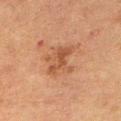follow-up: catalogued during a skin exam; not biopsied
lesion diameter: ≈4.5 mm
tile lighting: cross-polarized
imaging modality: ~15 mm tile from a whole-body skin photo
location: the abdomen
patient: female, about 50 years old
image-analysis metrics: a lesion area of about 11 mm² and an eccentricity of roughly 0.65; a lesion color around L≈44 a*≈20 b*≈30 in CIELAB, about 7 CIELAB-L* units darker than the surrounding skin, and a normalized border contrast of about 6; a border-irregularity index near 4.5/10, a within-lesion color-variation index near 4/10, and peripheral color asymmetry of about 1.5; an automated nevus-likeness rating near 0 out of 100